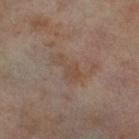Findings:
• follow-up: no biopsy performed (imaged during a skin exam)
• subject: female, aged around 55
• image source: 15 mm crop, total-body photography
• site: the right thigh
• lighting: cross-polarized illumination
• size: ~3.5 mm (longest diameter)